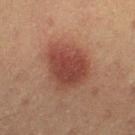<lesion>
<biopsy_status>not biopsied; imaged during a skin examination</biopsy_status>
<automated_metrics>
  <area_mm2_approx>20.0</area_mm2_approx>
  <eccentricity>0.55</eccentricity>
  <shape_asymmetry>0.25</shape_asymmetry>
  <border_irregularity_0_10>2.5</border_irregularity_0_10>
  <color_variation_0_10>3.5</color_variation_0_10>
  <peripheral_color_asymmetry>1.0</peripheral_color_asymmetry>
  <lesion_detection_confidence_0_100>100</lesion_detection_confidence_0_100>
</automated_metrics>
<patient>
  <sex>male</sex>
  <age_approx>60</age_approx>
</patient>
<image>
  <source>total-body photography crop</source>
  <field_of_view_mm>15</field_of_view_mm>
</image>
<lighting>cross-polarized</lighting>
<site>right thigh</site>
<lesion_size>
  <long_diameter_mm_approx>5.5</long_diameter_mm_approx>
</lesion_size>
</lesion>